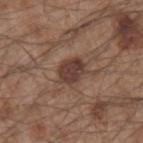Q: Was a biopsy performed?
A: catalogued during a skin exam; not biopsied
Q: What are the patient's age and sex?
A: male, aged approximately 55
Q: What is the lesion's diameter?
A: ≈3.5 mm
Q: What is the anatomic site?
A: the left forearm
Q: Automated lesion metrics?
A: border irregularity of about 2 on a 0–10 scale, a color-variation rating of about 2.5/10, and radial color variation of about 1
Q: How was the tile lit?
A: white-light
Q: How was this image acquired?
A: ~15 mm tile from a whole-body skin photo